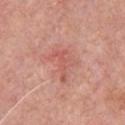Imaged during a routine full-body skin examination; the lesion was not biopsied and no histopathology is available. A male subject, aged 48–52. From the chest. Longest diameter approximately 4 mm. The tile uses white-light illumination. Cropped from a total-body skin-imaging series; the visible field is about 15 mm.